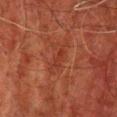Part of a total-body skin-imaging series; this lesion was reviewed on a skin check and was not flagged for biopsy. A male subject aged 58–62. A 15 mm close-up extracted from a 3D total-body photography capture. About 3 mm across. The lesion is located on the chest.